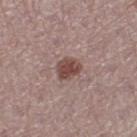The lesion was tiled from a total-body skin photograph and was not biopsied.
Measured at roughly 3 mm in maximum diameter.
A female subject aged approximately 40.
The lesion is located on the left thigh.
A lesion tile, about 15 mm wide, cut from a 3D total-body photograph.
The total-body-photography lesion software estimated border irregularity of about 2 on a 0–10 scale, internal color variation of about 3 on a 0–10 scale, and radial color variation of about 1.
This is a white-light tile.